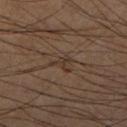<case>
  <biopsy_status>not biopsied; imaged during a skin examination</biopsy_status>
  <image>
    <source>total-body photography crop</source>
    <field_of_view_mm>15</field_of_view_mm>
  </image>
  <lesion_size>
    <long_diameter_mm_approx>3.0</long_diameter_mm_approx>
  </lesion_size>
  <patient>
    <sex>male</sex>
    <age_approx>50</age_approx>
  </patient>
  <site>right lower leg</site>
</case>